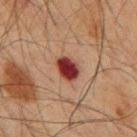follow-up — total-body-photography surveillance lesion; no biopsy
TBP lesion metrics — a lesion area of about 6 mm², a shape eccentricity near 0.65, and two-axis asymmetry of about 0.2; a mean CIELAB color near L≈31 a*≈26 b*≈23, roughly 17 lightness units darker than nearby skin, and a lesion-to-skin contrast of about 14.5 (normalized; higher = more distinct); a border-irregularity rating of about 1.5/10 and radial color variation of about 1; a classifier nevus-likeness of about 0/100 and a detector confidence of about 100 out of 100 that the crop contains a lesion
location — the mid back
subject — male, about 65 years old
lesion diameter — about 3 mm
acquisition — ~15 mm tile from a whole-body skin photo